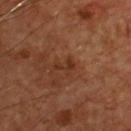notes: catalogued during a skin exam; not biopsied
patient: male, in their mid- to late 50s
imaging modality: ~15 mm tile from a whole-body skin photo
location: the front of the torso
automated lesion analysis: border irregularity of about 4.5 on a 0–10 scale and a color-variation rating of about 1/10; an automated nevus-likeness rating near 0 out of 100 and lesion-presence confidence of about 100/100
lesion size: ≈2.5 mm
illumination: cross-polarized